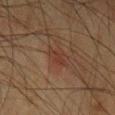Clinical impression: Captured during whole-body skin photography for melanoma surveillance; the lesion was not biopsied. Context: Imaged with cross-polarized lighting. From the left upper arm. The patient is a male approximately 75 years of age. Approximately 3 mm at its widest. This image is a 15 mm lesion crop taken from a total-body photograph. Automated tile analysis of the lesion measured a mean CIELAB color near L≈30 a*≈18 b*≈24 and a normalized lesion–skin contrast near 5.5. The analysis additionally found a classifier nevus-likeness of about 10/100 and a detector confidence of about 100 out of 100 that the crop contains a lesion.